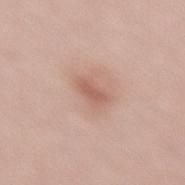Q: Was this lesion biopsied?
A: no biopsy performed (imaged during a skin exam)
Q: How large is the lesion?
A: about 2.5 mm
Q: What is the imaging modality?
A: ~15 mm tile from a whole-body skin photo
Q: What lighting was used for the tile?
A: white-light
Q: What is the anatomic site?
A: the lower back
Q: Who is the patient?
A: male, aged approximately 55